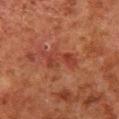Imaged during a routine full-body skin examination; the lesion was not biopsied and no histopathology is available. The lesion is on the right lower leg. This image is a 15 mm lesion crop taken from a total-body photograph. The patient is a male aged approximately 80.